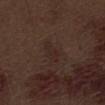notes = imaged on a skin check; not biopsied | location = the abdomen | acquisition = 15 mm crop, total-body photography | subject = male, aged around 70.